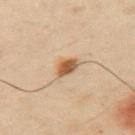On the left upper arm. Measured at roughly 2.5 mm in maximum diameter. Imaged with cross-polarized lighting. A region of skin cropped from a whole-body photographic capture, roughly 15 mm wide. A male patient in their mid-50s. The total-body-photography lesion software estimated an area of roughly 4 mm² and an eccentricity of roughly 0.65. The software also gave roughly 13 lightness units darker than nearby skin. It also reported lesion-presence confidence of about 100/100.